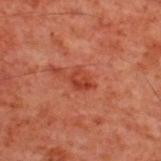A male subject approximately 60 years of age. The lesion is on the back. A lesion tile, about 15 mm wide, cut from a 3D total-body photograph. The lesion's longest dimension is about 3 mm. The tile uses cross-polarized illumination. The lesion-visualizer software estimated a detector confidence of about 100 out of 100 that the crop contains a lesion.